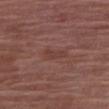| feature | finding |
|---|---|
| notes | total-body-photography surveillance lesion; no biopsy |
| patient | female, aged 68 to 72 |
| tile lighting | white-light |
| lesion diameter | about 2.5 mm |
| image source | 15 mm crop, total-body photography |
| anatomic site | the arm |